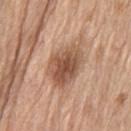Context: On the back. The recorded lesion diameter is about 5.5 mm. Cropped from a total-body skin-imaging series; the visible field is about 15 mm. Automated tile analysis of the lesion measured a lesion area of about 15 mm² and a symmetry-axis asymmetry near 0.25. The software also gave a border-irregularity index near 2.5/10, a color-variation rating of about 5.5/10, and a peripheral color-asymmetry measure near 1.5. This is a white-light tile. A male subject approximately 70 years of age.